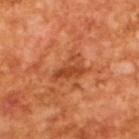This lesion was catalogued during total-body skin photography and was not selected for biopsy.
The tile uses cross-polarized illumination.
A male subject, aged 63 to 67.
A lesion tile, about 15 mm wide, cut from a 3D total-body photograph.
Longest diameter approximately 4 mm.
The total-body-photography lesion software estimated a classifier nevus-likeness of about 5/100 and a lesion-detection confidence of about 100/100.